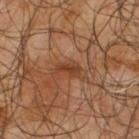* follow-up: imaged on a skin check; not biopsied
* size: about 2.5 mm
* patient: male, about 65 years old
* lighting: cross-polarized illumination
* location: the upper back
* image: total-body-photography crop, ~15 mm field of view
* image-analysis metrics: a footprint of about 3.5 mm², an eccentricity of roughly 0.9, and a symmetry-axis asymmetry near 0.3; about 8 CIELAB-L* units darker than the surrounding skin and a normalized lesion–skin contrast near 7.5